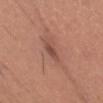- workup · imaged on a skin check; not biopsied
- patient · male, roughly 30 years of age
- anatomic site · the chest
- automated metrics · a footprint of about 2.5 mm², an outline eccentricity of about 0.95 (0 = round, 1 = elongated), and a symmetry-axis asymmetry near 0.3; a border-irregularity index near 3.5/10 and a within-lesion color-variation index near 0/10
- lighting · white-light
- diameter · ~3 mm (longest diameter)
- image source · ~15 mm crop, total-body skin-cancer survey